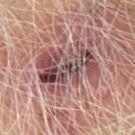Clinical impression:
Captured during whole-body skin photography for melanoma surveillance; the lesion was not biopsied.
Clinical summary:
From the left upper arm. A 15 mm crop from a total-body photograph taken for skin-cancer surveillance. The patient is a male aged 63 to 67. Approximately 7 mm at its widest.